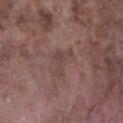biopsy_status: not biopsied; imaged during a skin examination
automated_metrics:
  cielab_L: 43
  cielab_a: 18
  cielab_b: 19
  vs_skin_darker_L: 6.0
  vs_skin_contrast_norm: 5.0
  border_irregularity_0_10: 7.5
  color_variation_0_10: 1.5
  peripheral_color_asymmetry: 0.5
  nevus_likeness_0_100: 0
  lesion_detection_confidence_0_100: 95
lighting: white-light
image:
  source: total-body photography crop
  field_of_view_mm: 15
lesion_size:
  long_diameter_mm_approx: 4.0
site: left lower leg
patient:
  sex: male
  age_approx: 75